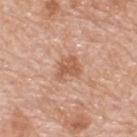Findings:
• notes — imaged on a skin check; not biopsied
• tile lighting — white-light
• size — about 3 mm
• TBP lesion metrics — a footprint of about 6 mm², an outline eccentricity of about 0.65 (0 = round, 1 = elongated), and two-axis asymmetry of about 0.4; an average lesion color of about L≈58 a*≈23 b*≈33 (CIELAB), about 10 CIELAB-L* units darker than the surrounding skin, and a normalized border contrast of about 7; a lesion-detection confidence of about 100/100
• patient — male, aged approximately 80
• imaging modality — ~15 mm tile from a whole-body skin photo
• body site — the upper back